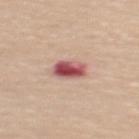{"biopsy_status": "not biopsied; imaged during a skin examination", "patient": {"sex": "female", "age_approx": 60}, "site": "upper back", "automated_metrics": {"cielab_L": 54, "cielab_a": 29, "cielab_b": 22, "vs_skin_darker_L": 17.0, "vs_skin_contrast_norm": 11.5, "border_irregularity_0_10": 2.0, "color_variation_0_10": 7.5, "nevus_likeness_0_100": 0, "lesion_detection_confidence_0_100": 100}, "lesion_size": {"long_diameter_mm_approx": 3.5}, "image": {"source": "total-body photography crop", "field_of_view_mm": 15}, "lighting": "white-light"}